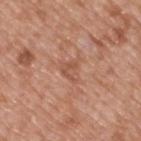Q: Was a biopsy performed?
A: total-body-photography surveillance lesion; no biopsy
Q: Automated lesion metrics?
A: an eccentricity of roughly 0.65 and a shape-asymmetry score of about 0.4 (0 = symmetric); a lesion color around L≈54 a*≈23 b*≈31 in CIELAB and a normalized border contrast of about 5; a nevus-likeness score of about 0/100 and lesion-presence confidence of about 100/100
Q: How large is the lesion?
A: about 2.5 mm
Q: What kind of image is this?
A: ~15 mm tile from a whole-body skin photo
Q: What are the patient's age and sex?
A: male, aged approximately 50
Q: Illumination type?
A: white-light
Q: What is the anatomic site?
A: the back